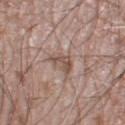Assessment: The lesion was tiled from a total-body skin photograph and was not biopsied.